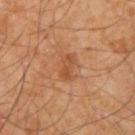This lesion was catalogued during total-body skin photography and was not selected for biopsy.
A male patient aged 58 to 62.
The tile uses cross-polarized illumination.
Cropped from a total-body skin-imaging series; the visible field is about 15 mm.
From the right upper arm.